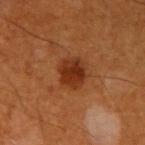Impression: No biopsy was performed on this lesion — it was imaged during a full skin examination and was not determined to be concerning.